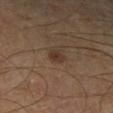– follow-up: imaged on a skin check; not biopsied
– image: total-body-photography crop, ~15 mm field of view
– lesion size: ~2 mm (longest diameter)
– subject: male, aged 58–62
– tile lighting: cross-polarized
– location: the left lower leg